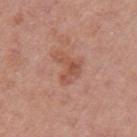The lesion was photographed on a routine skin check and not biopsied; there is no pathology result.
Automated tile analysis of the lesion measured a lesion color around L≈52 a*≈25 b*≈30 in CIELAB. The software also gave an automated nevus-likeness rating near 0 out of 100.
Longest diameter approximately 3.5 mm.
A lesion tile, about 15 mm wide, cut from a 3D total-body photograph.
The lesion is located on the arm.
A female patient, roughly 40 years of age.
The tile uses white-light illumination.